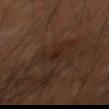Case summary:
- tile lighting · cross-polarized
- lesion size · about 3 mm
- image · ~15 mm tile from a whole-body skin photo
- site · the right thigh
- subject · male, roughly 65 years of age